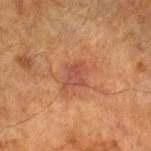Impression:
This lesion was catalogued during total-body skin photography and was not selected for biopsy.
Image and clinical context:
The patient is a male roughly 70 years of age. About 3 mm across. Captured under cross-polarized illumination. Located on the right lower leg. A 15 mm crop from a total-body photograph taken for skin-cancer surveillance.